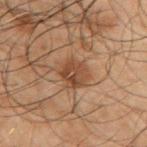Captured during whole-body skin photography for melanoma surveillance; the lesion was not biopsied. A male subject in their 50s. The tile uses cross-polarized illumination. Measured at roughly 4 mm in maximum diameter. On the right upper arm. A 15 mm close-up tile from a total-body photography series done for melanoma screening.